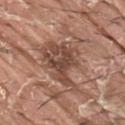This lesion was catalogued during total-body skin photography and was not selected for biopsy. A 15 mm close-up tile from a total-body photography series done for melanoma screening. A male patient roughly 40 years of age. The lesion is on the right upper arm.